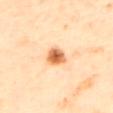Notes:
• follow-up · imaged on a skin check; not biopsied
• image · ~15 mm tile from a whole-body skin photo
• patient · male, about 65 years old
• tile lighting · cross-polarized
• lesion size · ~3 mm (longest diameter)
• location · the mid back